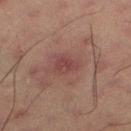Assessment: Part of a total-body skin-imaging series; this lesion was reviewed on a skin check and was not flagged for biopsy. Image and clinical context: A lesion tile, about 15 mm wide, cut from a 3D total-body photograph. The patient is a male approximately 60 years of age. About 2.5 mm across. Captured under cross-polarized illumination.